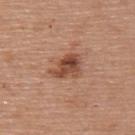<tbp_lesion>
  <biopsy_status>not biopsied; imaged during a skin examination</biopsy_status>
  <image>
    <source>total-body photography crop</source>
    <field_of_view_mm>15</field_of_view_mm>
  </image>
  <patient>
    <sex>female</sex>
    <age_approx>60</age_approx>
  </patient>
  <lighting>white-light</lighting>
  <site>upper back</site>
  <lesion_size>
    <long_diameter_mm_approx>4.0</long_diameter_mm_approx>
  </lesion_size>
</tbp_lesion>